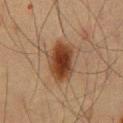notes: total-body-photography surveillance lesion; no biopsy
diameter: about 5.5 mm
image source: 15 mm crop, total-body photography
tile lighting: cross-polarized illumination
body site: the abdomen
patient: male, roughly 60 years of age